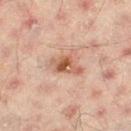The lesion was photographed on a routine skin check and not biopsied; there is no pathology result.
The lesion is on the right thigh.
Imaged with cross-polarized lighting.
The recorded lesion diameter is about 3.5 mm.
The subject is a male aged around 45.
A 15 mm close-up tile from a total-body photography series done for melanoma screening.